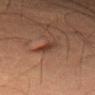Q: Is there a histopathology result?
A: total-body-photography surveillance lesion; no biopsy
Q: What is the anatomic site?
A: the right thigh
Q: What is the imaging modality?
A: ~15 mm crop, total-body skin-cancer survey
Q: What are the patient's age and sex?
A: male, aged 58–62